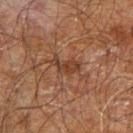Clinical impression:
No biopsy was performed on this lesion — it was imaged during a full skin examination and was not determined to be concerning.
Image and clinical context:
A region of skin cropped from a whole-body photographic capture, roughly 15 mm wide. The recorded lesion diameter is about 3.5 mm. A male patient aged around 70. Located on the right forearm.